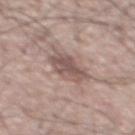biopsy_status: not biopsied; imaged during a skin examination
site: chest
lighting: white-light
image:
  source: total-body photography crop
  field_of_view_mm: 15
lesion_size:
  long_diameter_mm_approx: 5.0
patient:
  sex: male
  age_approx: 65